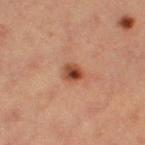<case>
  <biopsy_status>not biopsied; imaged during a skin examination</biopsy_status>
  <image>
    <source>total-body photography crop</source>
    <field_of_view_mm>15</field_of_view_mm>
  </image>
  <lighting>cross-polarized</lighting>
  <lesion_size>
    <long_diameter_mm_approx>2.5</long_diameter_mm_approx>
  </lesion_size>
  <site>left thigh</site>
  <patient>
    <sex>female</sex>
    <age_approx>40</age_approx>
  </patient>
</case>